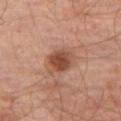  patient:
    sex: male
    age_approx: 70
  image:
    source: total-body photography crop
    field_of_view_mm: 15
  automated_metrics:
    area_mm2_approx: 7.0
    eccentricity: 0.4
    shape_asymmetry: 0.15
    border_irregularity_0_10: 1.5
    peripheral_color_asymmetry: 1.0
  lesion_size:
    long_diameter_mm_approx: 3.0
  lighting: cross-polarized
  site: left thigh